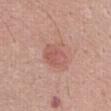Clinical impression: Part of a total-body skin-imaging series; this lesion was reviewed on a skin check and was not flagged for biopsy. Clinical summary: The lesion is located on the abdomen. About 3.5 mm across. Automated tile analysis of the lesion measured an area of roughly 10 mm² and an outline eccentricity of about 0.55 (0 = round, 1 = elongated). The analysis additionally found a color-variation rating of about 3/10 and a peripheral color-asymmetry measure near 1. The software also gave a nevus-likeness score of about 35/100. The subject is a male aged approximately 65. A close-up tile cropped from a whole-body skin photograph, about 15 mm across.